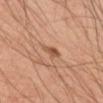No biopsy was performed on this lesion — it was imaged during a full skin examination and was not determined to be concerning.
This is a cross-polarized tile.
Measured at roughly 2 mm in maximum diameter.
This image is a 15 mm lesion crop taken from a total-body photograph.
The subject is a male about 45 years old.
Located on the left upper arm.
An algorithmic analysis of the crop reported a lesion area of about 2.5 mm², a shape eccentricity near 0.55, and two-axis asymmetry of about 0.35.